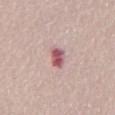| field | value |
|---|---|
| follow-up | no biopsy performed (imaged during a skin exam) |
| patient | male, roughly 65 years of age |
| diameter | about 3 mm |
| tile lighting | white-light illumination |
| anatomic site | the abdomen |
| imaging modality | ~15 mm crop, total-body skin-cancer survey |